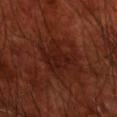<tbp_lesion>
  <biopsy_status>not biopsied; imaged during a skin examination</biopsy_status>
  <site>front of the torso</site>
  <patient>
    <sex>male</sex>
    <age_approx>70</age_approx>
  </patient>
  <image>
    <source>total-body photography crop</source>
    <field_of_view_mm>15</field_of_view_mm>
  </image>
  <lighting>cross-polarized</lighting>
</tbp_lesion>